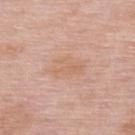Findings:
* notes — no biopsy performed (imaged during a skin exam)
* subject — female, about 50 years old
* acquisition — total-body-photography crop, ~15 mm field of view
* location — the upper back
* illumination — white-light illumination
* TBP lesion metrics — an average lesion color of about L≈64 a*≈20 b*≈31 (CIELAB), a lesion–skin lightness drop of about 5, and a normalized border contrast of about 4.5; border irregularity of about 2 on a 0–10 scale
* size — ≈4.5 mm